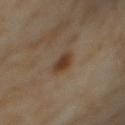Assessment:
Recorded during total-body skin imaging; not selected for excision or biopsy.
Clinical summary:
The lesion is located on the mid back. The patient is a female aged around 55. Cropped from a total-body skin-imaging series; the visible field is about 15 mm. Approximately 2.5 mm at its widest.